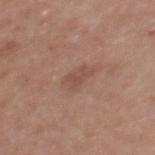Impression:
The lesion was photographed on a routine skin check and not biopsied; there is no pathology result.
Image and clinical context:
A female subject in their 40s. This is a white-light tile. The lesion's longest dimension is about 3 mm. A lesion tile, about 15 mm wide, cut from a 3D total-body photograph. The lesion is located on the back. The lesion-visualizer software estimated roughly 7 lightness units darker than nearby skin and a lesion-to-skin contrast of about 5 (normalized; higher = more distinct). It also reported a border-irregularity index near 3/10 and a within-lesion color-variation index near 2/10.